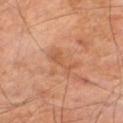Clinical impression: Recorded during total-body skin imaging; not selected for excision or biopsy. Background: Cropped from a total-body skin-imaging series; the visible field is about 15 mm. The tile uses cross-polarized illumination. A male subject, aged around 70. The lesion is located on the right thigh. About 4 mm across.